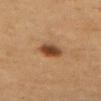Impression: The lesion was tiled from a total-body skin photograph and was not biopsied. Background: From the left upper arm. A female subject aged around 45. A 15 mm crop from a total-body photograph taken for skin-cancer surveillance.